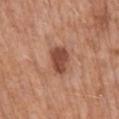Impression:
The lesion was tiled from a total-body skin photograph and was not biopsied.
Acquisition and patient details:
A 15 mm close-up extracted from a 3D total-body photography capture. The subject is a male approximately 70 years of age. The lesion is on the mid back.